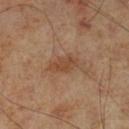Clinical impression: Recorded during total-body skin imaging; not selected for excision or biopsy. Clinical summary: A roughly 15 mm field-of-view crop from a total-body skin photograph. This is a cross-polarized tile. An algorithmic analysis of the crop reported a mean CIELAB color near L≈44 a*≈20 b*≈31, a lesion–skin lightness drop of about 8, and a normalized lesion–skin contrast near 6.5. It also reported a color-variation rating of about 1.5/10 and radial color variation of about 0.5. The lesion's longest dimension is about 3.5 mm. The lesion is located on the left lower leg. The patient is a male roughly 70 years of age.